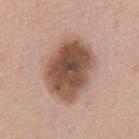Q: Was a biopsy performed?
A: imaged on a skin check; not biopsied
Q: What is the anatomic site?
A: the mid back
Q: Illumination type?
A: white-light
Q: Lesion size?
A: ~7.5 mm (longest diameter)
Q: Automated lesion metrics?
A: a within-lesion color-variation index near 7/10; a classifier nevus-likeness of about 10/100 and a lesion-detection confidence of about 100/100
Q: Patient demographics?
A: male, in their mid- to late 50s
Q: What is the imaging modality?
A: ~15 mm tile from a whole-body skin photo